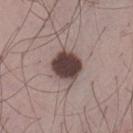This lesion was catalogued during total-body skin photography and was not selected for biopsy.
Cropped from a whole-body photographic skin survey; the tile spans about 15 mm.
Longest diameter approximately 4 mm.
The patient is a male approximately 35 years of age.
Located on the left thigh.